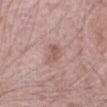Impression: Captured during whole-body skin photography for melanoma surveillance; the lesion was not biopsied. Background: The lesion is on the front of the torso. The lesion's longest dimension is about 3 mm. A male patient aged 68–72. The total-body-photography lesion software estimated a lesion color around L≈56 a*≈20 b*≈25 in CIELAB, roughly 8 lightness units darker than nearby skin, and a lesion-to-skin contrast of about 6 (normalized; higher = more distinct). The analysis additionally found internal color variation of about 2.5 on a 0–10 scale and peripheral color asymmetry of about 0.5. The software also gave a nevus-likeness score of about 40/100 and a lesion-detection confidence of about 100/100. This image is a 15 mm lesion crop taken from a total-body photograph. Captured under white-light illumination.